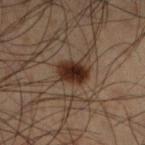Context:
A male patient, in their 50s. A lesion tile, about 15 mm wide, cut from a 3D total-body photograph. On the left lower leg. The lesion's longest dimension is about 3.5 mm. This is a cross-polarized tile.